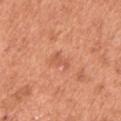From the left upper arm.
A male subject aged approximately 65.
Measured at roughly 2.5 mm in maximum diameter.
The total-body-photography lesion software estimated a lesion area of about 2.5 mm², an outline eccentricity of about 0.85 (0 = round, 1 = elongated), and two-axis asymmetry of about 0.55. It also reported a border-irregularity index near 6/10 and internal color variation of about 0 on a 0–10 scale.
A close-up tile cropped from a whole-body skin photograph, about 15 mm across.